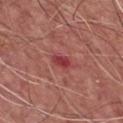<lesion>
  <biopsy_status>not biopsied; imaged during a skin examination</biopsy_status>
  <lesion_size>
    <long_diameter_mm_approx>3.0</long_diameter_mm_approx>
  </lesion_size>
  <image>
    <source>total-body photography crop</source>
    <field_of_view_mm>15</field_of_view_mm>
  </image>
  <site>chest</site>
  <automated_metrics>
    <cielab_L>42</cielab_L>
    <cielab_a>35</cielab_a>
    <cielab_b>22</cielab_b>
    <vs_skin_contrast_norm>7.5</vs_skin_contrast_norm>
    <peripheral_color_asymmetry>1.0</peripheral_color_asymmetry>
    <nevus_likeness_0_100>0</nevus_likeness_0_100>
    <lesion_detection_confidence_0_100>100</lesion_detection_confidence_0_100>
  </automated_metrics>
  <patient>
    <sex>male</sex>
    <age_approx>75</age_approx>
  </patient>
</lesion>